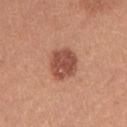{
  "automated_metrics": {
    "cielab_L": 50,
    "cielab_a": 26,
    "cielab_b": 30,
    "vs_skin_darker_L": 13.0,
    "vs_skin_contrast_norm": 9.0,
    "lesion_detection_confidence_0_100": 100
  },
  "lighting": "white-light",
  "image": {
    "source": "total-body photography crop",
    "field_of_view_mm": 15
  },
  "lesion_size": {
    "long_diameter_mm_approx": 3.5
  },
  "patient": {
    "sex": "female",
    "age_approx": 25
  },
  "site": "left upper arm"
}